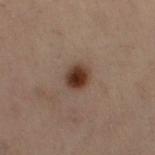Context: A 15 mm crop from a total-body photograph taken for skin-cancer surveillance. The tile uses cross-polarized illumination. Located on the front of the torso. A female subject aged 53 to 57.